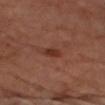This lesion was catalogued during total-body skin photography and was not selected for biopsy.
The total-body-photography lesion software estimated a footprint of about 2.5 mm² and two-axis asymmetry of about 0.25. The software also gave about 8 CIELAB-L* units darker than the surrounding skin and a lesion-to-skin contrast of about 8 (normalized; higher = more distinct).
A male patient in their 70s.
A close-up tile cropped from a whole-body skin photograph, about 15 mm across.
The lesion's longest dimension is about 2.5 mm.
The tile uses cross-polarized illumination.
From the right upper arm.